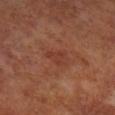Part of a total-body skin-imaging series; this lesion was reviewed on a skin check and was not flagged for biopsy. A male patient roughly 70 years of age. An algorithmic analysis of the crop reported a shape-asymmetry score of about 0.35 (0 = symmetric). And it measured border irregularity of about 3 on a 0–10 scale, internal color variation of about 2 on a 0–10 scale, and a peripheral color-asymmetry measure near 0.5. On the right lower leg. A roughly 15 mm field-of-view crop from a total-body skin photograph. The lesion's longest dimension is about 3 mm. Imaged with cross-polarized lighting.